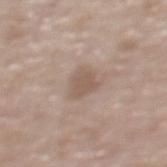Assessment:
This lesion was catalogued during total-body skin photography and was not selected for biopsy.
Background:
A male subject aged 83 or older. The lesion is on the upper back. A roughly 15 mm field-of-view crop from a total-body skin photograph.